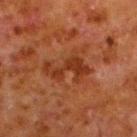Assessment:
Part of a total-body skin-imaging series; this lesion was reviewed on a skin check and was not flagged for biopsy.
Context:
The tile uses cross-polarized illumination. From the left lower leg. A male subject, aged 78 to 82. Automated tile analysis of the lesion measured a mean CIELAB color near L≈28 a*≈23 b*≈30, about 7 CIELAB-L* units darker than the surrounding skin, and a lesion-to-skin contrast of about 7.5 (normalized; higher = more distinct). A 15 mm close-up extracted from a 3D total-body photography capture.